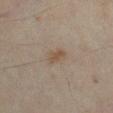Imaged during a routine full-body skin examination; the lesion was not biopsied and no histopathology is available.
Automated tile analysis of the lesion measured a footprint of about 3.5 mm², an eccentricity of roughly 0.85, and two-axis asymmetry of about 0.3. It also reported a lesion color around L≈42 a*≈12 b*≈24 in CIELAB and a normalized border contrast of about 6.5. And it measured an automated nevus-likeness rating near 20 out of 100 and a detector confidence of about 100 out of 100 that the crop contains a lesion.
Longest diameter approximately 2.5 mm.
A region of skin cropped from a whole-body photographic capture, roughly 15 mm wide.
Captured under cross-polarized illumination.
A male subject, approximately 45 years of age.
Located on the leg.